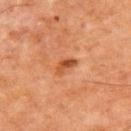This lesion was catalogued during total-body skin photography and was not selected for biopsy. On the upper back. This is a cross-polarized tile. Cropped from a total-body skin-imaging series; the visible field is about 15 mm. Approximately 3 mm at its widest. The patient is a male aged around 65.